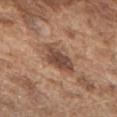Impression: Captured during whole-body skin photography for melanoma surveillance; the lesion was not biopsied. Clinical summary: This image is a 15 mm lesion crop taken from a total-body photograph. From the chest. Captured under white-light illumination. A male patient approximately 75 years of age. The lesion's longest dimension is about 5.5 mm.